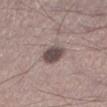{"image": {"source": "total-body photography crop", "field_of_view_mm": 15}, "patient": {"sex": "male", "age_approx": 45}, "lesion_size": {"long_diameter_mm_approx": 4.0}, "site": "left lower leg", "automated_metrics": {"area_mm2_approx": 10.0, "eccentricity": 0.65, "shape_asymmetry": 0.2, "lesion_detection_confidence_0_100": 100}}